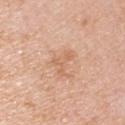| feature | finding |
|---|---|
| biopsy status | catalogued during a skin exam; not biopsied |
| image source | ~15 mm crop, total-body skin-cancer survey |
| tile lighting | white-light illumination |
| lesion size | ~3.5 mm (longest diameter) |
| patient | male, about 50 years old |
| location | the right upper arm |